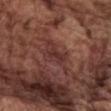| feature | finding |
|---|---|
| follow-up | catalogued during a skin exam; not biopsied |
| lighting | white-light |
| body site | the chest |
| subject | male, in their mid-70s |
| acquisition | total-body-photography crop, ~15 mm field of view |
| lesion size | about 5 mm |